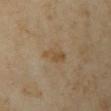Q: Was this lesion biopsied?
A: total-body-photography surveillance lesion; no biopsy
Q: Patient demographics?
A: female, about 35 years old
Q: What is the imaging modality?
A: total-body-photography crop, ~15 mm field of view
Q: Lesion location?
A: the left upper arm